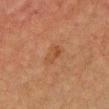The lesion was tiled from a total-body skin photograph and was not biopsied.
Automated tile analysis of the lesion measured a shape eccentricity near 0.9 and two-axis asymmetry of about 0.35. It also reported internal color variation of about 1 on a 0–10 scale and a peripheral color-asymmetry measure near 0.5. The software also gave a classifier nevus-likeness of about 0/100 and a lesion-detection confidence of about 100/100.
A male patient aged approximately 60.
Longest diameter approximately 3 mm.
Imaged with cross-polarized lighting.
A 15 mm close-up extracted from a 3D total-body photography capture.
On the front of the torso.